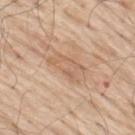- workup: no biopsy performed (imaged during a skin exam)
- image source: total-body-photography crop, ~15 mm field of view
- anatomic site: the upper back
- tile lighting: white-light illumination
- lesion diameter: about 4.5 mm
- patient: male, approximately 70 years of age